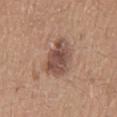The lesion was photographed on a routine skin check and not biopsied; there is no pathology result.
A region of skin cropped from a whole-body photographic capture, roughly 15 mm wide.
The recorded lesion diameter is about 5 mm.
Imaged with white-light lighting.
Located on the mid back.
The subject is a male about 20 years old.